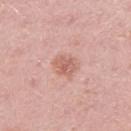– follow-up: no biopsy performed (imaged during a skin exam)
– tile lighting: white-light
– site: the right upper arm
– size: ~3 mm (longest diameter)
– patient: female, aged approximately 30
– image-analysis metrics: a footprint of about 5 mm², an eccentricity of roughly 0.5, and a symmetry-axis asymmetry near 0.2; a color-variation rating of about 3/10 and peripheral color asymmetry of about 1; an automated nevus-likeness rating near 25 out of 100 and lesion-presence confidence of about 100/100
– image source: ~15 mm tile from a whole-body skin photo